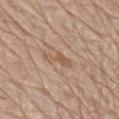Q: Is there a histopathology result?
A: total-body-photography surveillance lesion; no biopsy
Q: What is the imaging modality?
A: 15 mm crop, total-body photography
Q: What lighting was used for the tile?
A: white-light
Q: Patient demographics?
A: male, aged 78 to 82
Q: How large is the lesion?
A: about 4 mm
Q: Where on the body is the lesion?
A: the mid back